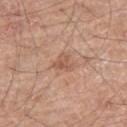{"image": {"source": "total-body photography crop", "field_of_view_mm": 15}, "site": "right thigh", "patient": {"sex": "male", "age_approx": 70}}